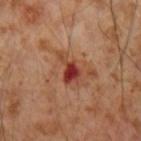| field | value |
|---|---|
| biopsy status | no biopsy performed (imaged during a skin exam) |
| acquisition | ~15 mm tile from a whole-body skin photo |
| patient | male, aged 53–57 |
| location | the right forearm |